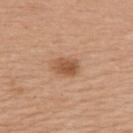Impression:
No biopsy was performed on this lesion — it was imaged during a full skin examination and was not determined to be concerning.
Background:
An algorithmic analysis of the crop reported a lesion color around L≈53 a*≈22 b*≈34 in CIELAB, a lesion–skin lightness drop of about 11, and a normalized lesion–skin contrast near 8. The lesion is on the back. The patient is a female aged approximately 60. A 15 mm close-up extracted from a 3D total-body photography capture. Measured at roughly 3 mm in maximum diameter.